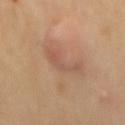No biopsy was performed on this lesion — it was imaged during a full skin examination and was not determined to be concerning. On the back. The recorded lesion diameter is about 4 mm. The patient is a female aged approximately 55. Cropped from a total-body skin-imaging series; the visible field is about 15 mm.